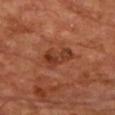No biopsy was performed on this lesion — it was imaged during a full skin examination and was not determined to be concerning.
The lesion-visualizer software estimated a footprint of about 7 mm² and a symmetry-axis asymmetry near 0.4.
A 15 mm close-up tile from a total-body photography series done for melanoma screening.
About 4 mm across.
From the chest.
A male subject, in their 70s.